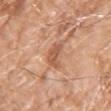This lesion was catalogued during total-body skin photography and was not selected for biopsy. A male subject roughly 80 years of age. On the left upper arm. Cropped from a total-body skin-imaging series; the visible field is about 15 mm. Measured at roughly 4 mm in maximum diameter.